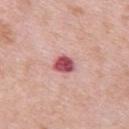{
  "biopsy_status": "not biopsied; imaged during a skin examination",
  "lighting": "white-light",
  "lesion_size": {
    "long_diameter_mm_approx": 2.5
  },
  "image": {
    "source": "total-body photography crop",
    "field_of_view_mm": 15
  },
  "patient": {
    "sex": "female",
    "age_approx": 65
  },
  "site": "upper back"
}